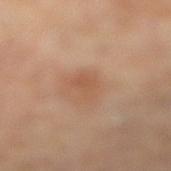{
  "biopsy_status": "not biopsied; imaged during a skin examination",
  "site": "left leg",
  "image": {
    "source": "total-body photography crop",
    "field_of_view_mm": 15
  },
  "lesion_size": {
    "long_diameter_mm_approx": 3.0
  },
  "patient": {
    "sex": "female",
    "age_approx": 65
  },
  "automated_metrics": {
    "area_mm2_approx": 4.5,
    "eccentricity": 0.75,
    "shape_asymmetry": 0.4,
    "vs_skin_darker_L": 6.0,
    "vs_skin_contrast_norm": 5.0
  },
  "lighting": "cross-polarized"
}